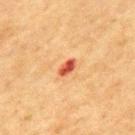Clinical impression: The lesion was tiled from a total-body skin photograph and was not biopsied. Clinical summary: The subject is a male aged around 75. Longest diameter approximately 2.5 mm. The tile uses cross-polarized illumination. From the mid back. A 15 mm crop from a total-body photograph taken for skin-cancer surveillance.